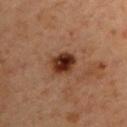{"biopsy_status": "not biopsied; imaged during a skin examination", "site": "right upper arm", "image": {"source": "total-body photography crop", "field_of_view_mm": 15}, "patient": {"sex": "male", "age_approx": 60}}